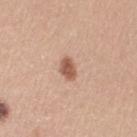<tbp_lesion>
  <site>arm</site>
  <lighting>white-light</lighting>
  <lesion_size>
    <long_diameter_mm_approx>2.5</long_diameter_mm_approx>
  </lesion_size>
  <patient>
    <sex>female</sex>
    <age_approx>30</age_approx>
  </patient>
  <automated_metrics>
    <area_mm2_approx>4.0</area_mm2_approx>
    <eccentricity>0.7</eccentricity>
    <vs_skin_darker_L>14.0</vs_skin_darker_L>
    <vs_skin_contrast_norm>9.0</vs_skin_contrast_norm>
  </automated_metrics>
  <image>
    <source>total-body photography crop</source>
    <field_of_view_mm>15</field_of_view_mm>
  </image>
</tbp_lesion>